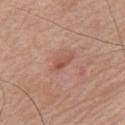biopsy status = imaged on a skin check; not biopsied | illumination = white-light illumination | diameter = about 3 mm | subject = male, aged 73 to 77 | image = ~15 mm crop, total-body skin-cancer survey | site = the chest.